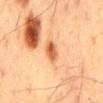The lesion was photographed on a routine skin check and not biopsied; there is no pathology result.
The lesion is on the mid back.
Measured at roughly 2.5 mm in maximum diameter.
Imaged with cross-polarized lighting.
The total-body-photography lesion software estimated a border-irregularity rating of about 1.5/10 and a color-variation rating of about 4.5/10.
A 15 mm close-up extracted from a 3D total-body photography capture.
The patient is a male aged 58 to 62.